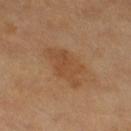  biopsy_status: not biopsied; imaged during a skin examination
  image:
    source: total-body photography crop
    field_of_view_mm: 15
  lesion_size:
    long_diameter_mm_approx: 5.5
  automated_metrics:
    eccentricity: 0.8
    shape_asymmetry: 0.25
    border_irregularity_0_10: 3.0
    color_variation_0_10: 2.5
    peripheral_color_asymmetry: 1.0
    nevus_likeness_0_100: 10
    lesion_detection_confidence_0_100: 100
  patient:
    sex: female
  lighting: cross-polarized